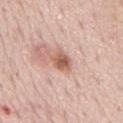<record>
<biopsy_status>not biopsied; imaged during a skin examination</biopsy_status>
<lesion_size>
  <long_diameter_mm_approx>3.0</long_diameter_mm_approx>
</lesion_size>
<automated_metrics>
  <shape_asymmetry>0.2</shape_asymmetry>
  <cielab_L>59</cielab_L>
  <cielab_a>22</cielab_a>
  <cielab_b>28</cielab_b>
  <color_variation_0_10>3.5</color_variation_0_10>
  <peripheral_color_asymmetry>1.0</peripheral_color_asymmetry>
</automated_metrics>
<lighting>white-light</lighting>
<patient>
  <sex>male</sex>
  <age_approx>75</age_approx>
</patient>
<site>mid back</site>
<image>
  <source>total-body photography crop</source>
  <field_of_view_mm>15</field_of_view_mm>
</image>
</record>